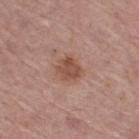size — ~3 mm (longest diameter); tile lighting — white-light illumination; subject — female, aged 63 to 67; site — the right thigh; image source — ~15 mm tile from a whole-body skin photo.